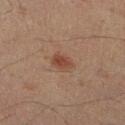Q: Was a biopsy performed?
A: no biopsy performed (imaged during a skin exam)
Q: What are the patient's age and sex?
A: male, in their 50s
Q: Where on the body is the lesion?
A: the right lower leg
Q: What is the imaging modality?
A: ~15 mm tile from a whole-body skin photo